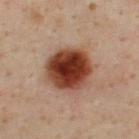Notes:
• workup: imaged on a skin check; not biopsied
• size: ~5.5 mm (longest diameter)
• patient: male, aged approximately 45
• site: the back
• automated metrics: a footprint of about 21 mm², an outline eccentricity of about 0.5 (0 = round, 1 = elongated), and a symmetry-axis asymmetry near 0.1; a lesion color around L≈39 a*≈25 b*≈30 in CIELAB, a lesion–skin lightness drop of about 20, and a lesion-to-skin contrast of about 15 (normalized; higher = more distinct); an automated nevus-likeness rating near 100 out of 100
• acquisition: ~15 mm tile from a whole-body skin photo
• lighting: cross-polarized